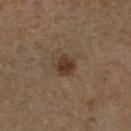This lesion was catalogued during total-body skin photography and was not selected for biopsy. The subject is a male roughly 65 years of age. The lesion is on the right lower leg. A region of skin cropped from a whole-body photographic capture, roughly 15 mm wide.